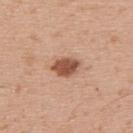biopsy status=no biopsy performed (imaged during a skin exam)
tile lighting=white-light illumination
lesion size=≈3.5 mm
site=the upper back
patient=male, approximately 30 years of age
image-analysis metrics=a border-irregularity index near 1.5/10 and a color-variation rating of about 2.5/10; a classifier nevus-likeness of about 95/100
image=~15 mm tile from a whole-body skin photo